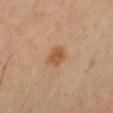The lesion is on the leg.
This is a cross-polarized tile.
A close-up tile cropped from a whole-body skin photograph, about 15 mm across.
A male patient, aged approximately 60.
Automated image analysis of the tile measured a lesion area of about 4.5 mm². And it measured a border-irregularity index near 2.5/10, internal color variation of about 2 on a 0–10 scale, and a peripheral color-asymmetry measure near 0.5.